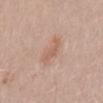| feature | finding |
|---|---|
| biopsy status | catalogued during a skin exam; not biopsied |
| subject | female, aged approximately 35 |
| illumination | white-light illumination |
| automated metrics | internal color variation of about 1.5 on a 0–10 scale and a peripheral color-asymmetry measure near 0.5 |
| size | about 3.5 mm |
| acquisition | ~15 mm crop, total-body skin-cancer survey |
| location | the lower back |